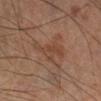This lesion was catalogued during total-body skin photography and was not selected for biopsy. The lesion's longest dimension is about 4.5 mm. A male patient approximately 60 years of age. Automated image analysis of the tile measured a mean CIELAB color near L≈41 a*≈19 b*≈28 and a lesion-to-skin contrast of about 5.5 (normalized; higher = more distinct). It also reported border irregularity of about 7.5 on a 0–10 scale, a within-lesion color-variation index near 2.5/10, and peripheral color asymmetry of about 0.5. It also reported a nevus-likeness score of about 0/100 and lesion-presence confidence of about 100/100. Located on the right lower leg. A roughly 15 mm field-of-view crop from a total-body skin photograph. This is a cross-polarized tile.